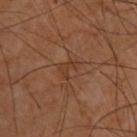No biopsy was performed on this lesion — it was imaged during a full skin examination and was not determined to be concerning. The subject is a male aged 58 to 62. A region of skin cropped from a whole-body photographic capture, roughly 15 mm wide. Imaged with cross-polarized lighting. Approximately 3 mm at its widest. Located on the upper back.